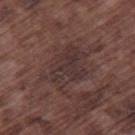Findings:
• notes: catalogued during a skin exam; not biopsied
• image: total-body-photography crop, ~15 mm field of view
• anatomic site: the right thigh
• subject: male, aged approximately 75
• lesion diameter: ≈6 mm
• automated metrics: an area of roughly 16 mm² and an eccentricity of roughly 0.75; a mean CIELAB color near L≈32 a*≈16 b*≈17 and about 6 CIELAB-L* units darker than the surrounding skin; border irregularity of about 4 on a 0–10 scale, a color-variation rating of about 3/10, and peripheral color asymmetry of about 1
• tile lighting: white-light illumination